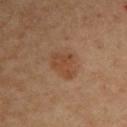Clinical impression: The lesion was tiled from a total-body skin photograph and was not biopsied. Clinical summary: Approximately 4 mm at its widest. A female subject aged 43 to 47. On the right upper arm. A 15 mm close-up tile from a total-body photography series done for melanoma screening.